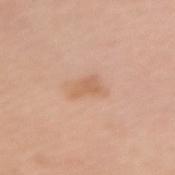Impression: This lesion was catalogued during total-body skin photography and was not selected for biopsy. Image and clinical context: About 3.5 mm across. Automated tile analysis of the lesion measured an automated nevus-likeness rating near 0 out of 100 and a lesion-detection confidence of about 100/100. The lesion is on the left forearm. The patient is a female about 65 years old. Cropped from a total-body skin-imaging series; the visible field is about 15 mm.